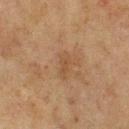Q: Was this lesion biopsied?
A: no biopsy performed (imaged during a skin exam)
Q: Lesion location?
A: the chest
Q: What lighting was used for the tile?
A: cross-polarized illumination
Q: What kind of image is this?
A: ~15 mm tile from a whole-body skin photo
Q: What is the lesion's diameter?
A: ~3 mm (longest diameter)
Q: Who is the patient?
A: male, approximately 75 years of age